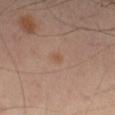Part of a total-body skin-imaging series; this lesion was reviewed on a skin check and was not flagged for biopsy.
A lesion tile, about 15 mm wide, cut from a 3D total-body photograph.
On the left thigh.
The subject is a male approximately 65 years of age.